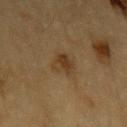The tile uses cross-polarized illumination. The recorded lesion diameter is about 3 mm. On the left upper arm. A male patient aged 83 or over. An algorithmic analysis of the crop reported an average lesion color of about L≈32 a*≈14 b*≈29 (CIELAB) and a normalized lesion–skin contrast near 7.5. And it measured a border-irregularity rating of about 3.5/10 and a color-variation rating of about 2/10. And it measured a nevus-likeness score of about 75/100 and a lesion-detection confidence of about 100/100. A region of skin cropped from a whole-body photographic capture, roughly 15 mm wide.